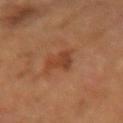Part of a total-body skin-imaging series; this lesion was reviewed on a skin check and was not flagged for biopsy.
Located on the right forearm.
A female patient, in their 60s.
A 15 mm crop from a total-body photograph taken for skin-cancer surveillance.
About 3 mm across.